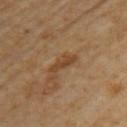* workup — catalogued during a skin exam; not biopsied
* image-analysis metrics — a lesion area of about 3.5 mm² and a shape eccentricity near 0.95
* patient — female, in their mid- to late 60s
* illumination — cross-polarized
* diameter — ~3.5 mm (longest diameter)
* location — the left upper arm
* image — total-body-photography crop, ~15 mm field of view